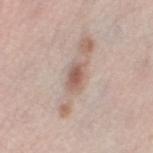Image and clinical context:
On the left thigh. The lesion-visualizer software estimated a lesion area of about 5.5 mm² and a shape-asymmetry score of about 0.25 (0 = symmetric). The software also gave a border-irregularity index near 2.5/10 and radial color variation of about 1. The analysis additionally found a nevus-likeness score of about 35/100 and a detector confidence of about 100 out of 100 that the crop contains a lesion. The subject is a female about 65 years old. About 3.5 mm across. Captured under white-light illumination. A 15 mm close-up extracted from a 3D total-body photography capture.